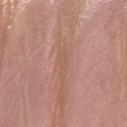Q: Was a biopsy performed?
A: total-body-photography surveillance lesion; no biopsy
Q: What did automated image analysis measure?
A: an area of roughly 3.5 mm², an eccentricity of roughly 0.95, and a shape-asymmetry score of about 0.5 (0 = symmetric); border irregularity of about 6 on a 0–10 scale, a within-lesion color-variation index near 0.5/10, and a peripheral color-asymmetry measure near 0; a classifier nevus-likeness of about 0/100 and a detector confidence of about 75 out of 100 that the crop contains a lesion
Q: What lighting was used for the tile?
A: white-light
Q: How large is the lesion?
A: ≈4 mm
Q: Who is the patient?
A: female, aged approximately 40
Q: What kind of image is this?
A: ~15 mm crop, total-body skin-cancer survey
Q: Lesion location?
A: the right lower leg